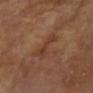Imaged during a routine full-body skin examination; the lesion was not biopsied and no histopathology is available.
The lesion is on the arm.
About 4 mm across.
Cropped from a total-body skin-imaging series; the visible field is about 15 mm.
The patient is a female aged 73–77.